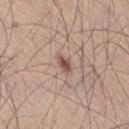Assessment: This lesion was catalogued during total-body skin photography and was not selected for biopsy. Image and clinical context: The lesion is on the right thigh. The patient is a male in their mid- to late 50s. A region of skin cropped from a whole-body photographic capture, roughly 15 mm wide. An algorithmic analysis of the crop reported a lesion area of about 3 mm² and an eccentricity of roughly 0.8. And it measured a mean CIELAB color near L≈52 a*≈19 b*≈25, roughly 12 lightness units darker than nearby skin, and a normalized border contrast of about 8.5. The software also gave a classifier nevus-likeness of about 95/100 and a lesion-detection confidence of about 100/100.